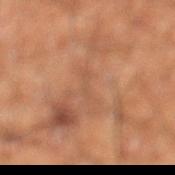{"biopsy_status": "not biopsied; imaged during a skin examination", "lighting": "cross-polarized", "image": {"source": "total-body photography crop", "field_of_view_mm": 15}, "patient": {"sex": "male", "age_approx": 60}, "site": "left lower leg", "lesion_size": {"long_diameter_mm_approx": 3.5}}